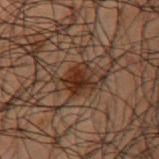Imaged during a routine full-body skin examination; the lesion was not biopsied and no histopathology is available. A male subject, about 50 years old. Cropped from a total-body skin-imaging series; the visible field is about 15 mm. Approximately 3.5 mm at its widest. This is a cross-polarized tile. Automated image analysis of the tile measured a lesion color around L≈22 a*≈15 b*≈21 in CIELAB and a normalized border contrast of about 9. Located on the left upper arm.